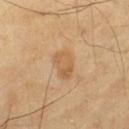Findings:
* workup · imaged on a skin check; not biopsied
* image · 15 mm crop, total-body photography
* patient · male, approximately 70 years of age
* anatomic site · the left thigh
* lesion diameter · about 3.5 mm
* illumination · cross-polarized